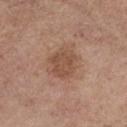| field | value |
|---|---|
| follow-up | catalogued during a skin exam; not biopsied |
| anatomic site | the left forearm |
| patient | female, in their mid- to late 60s |
| imaging modality | total-body-photography crop, ~15 mm field of view |
| diameter | ≈4 mm |
| lighting | white-light illumination |
| automated lesion analysis | a lesion area of about 9 mm², a shape eccentricity near 0.65, and two-axis asymmetry of about 0.2; a normalized lesion–skin contrast near 6.5; a nevus-likeness score of about 10/100 |